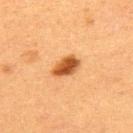{"biopsy_status": "not biopsied; imaged during a skin examination", "lesion_size": {"long_diameter_mm_approx": 3.5}, "image": {"source": "total-body photography crop", "field_of_view_mm": 15}, "site": "upper back", "lighting": "cross-polarized", "patient": {"sex": "female", "age_approx": 40}}